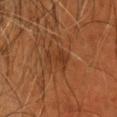notes: imaged on a skin check; not biopsied | location: the head or neck | image: total-body-photography crop, ~15 mm field of view | patient: male, aged 53 to 57 | lesion size: about 3.5 mm.